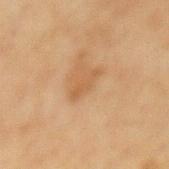Imaged during a routine full-body skin examination; the lesion was not biopsied and no histopathology is available. From the back. A female patient, approximately 55 years of age. About 3.5 mm across. The total-body-photography lesion software estimated a mean CIELAB color near L≈50 a*≈19 b*≈35, roughly 7 lightness units darker than nearby skin, and a lesion-to-skin contrast of about 5.5 (normalized; higher = more distinct). And it measured a border-irregularity index near 4.5/10, a within-lesion color-variation index near 0/10, and a peripheral color-asymmetry measure near 0. It also reported a nevus-likeness score of about 0/100 and a lesion-detection confidence of about 100/100. A close-up tile cropped from a whole-body skin photograph, about 15 mm across.